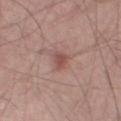Case summary:
* biopsy status: total-body-photography surveillance lesion; no biopsy
* patient: male, aged 53–57
* diameter: ~2.5 mm (longest diameter)
* illumination: white-light illumination
* anatomic site: the leg
* image: ~15 mm tile from a whole-body skin photo
* image-analysis metrics: two-axis asymmetry of about 0.35; a border-irregularity rating of about 3.5/10, a color-variation rating of about 1/10, and peripheral color asymmetry of about 0.5; lesion-presence confidence of about 100/100